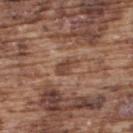notes: total-body-photography surveillance lesion; no biopsy | image source: 15 mm crop, total-body photography | anatomic site: the upper back | subject: male, aged 73–77 | tile lighting: white-light | TBP lesion metrics: a shape eccentricity near 0.8; a mean CIELAB color near L≈45 a*≈20 b*≈28, about 9 CIELAB-L* units darker than the surrounding skin, and a lesion-to-skin contrast of about 7 (normalized; higher = more distinct); a border-irregularity rating of about 3/10, a color-variation rating of about 3.5/10, and a peripheral color-asymmetry measure near 1.5.